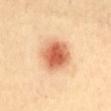The lesion was photographed on a routine skin check and not biopsied; there is no pathology result. A female patient aged 38–42. About 4.5 mm across. On the mid back. A 15 mm close-up extracted from a 3D total-body photography capture. Automated image analysis of the tile measured a footprint of about 13 mm², a shape eccentricity near 0.65, and a symmetry-axis asymmetry near 0.15. The analysis additionally found a mean CIELAB color near L≈64 a*≈29 b*≈39, about 17 CIELAB-L* units darker than the surrounding skin, and a normalized lesion–skin contrast near 10. The software also gave a classifier nevus-likeness of about 100/100 and a detector confidence of about 100 out of 100 that the crop contains a lesion.